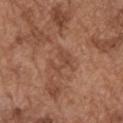notes: imaged on a skin check; not biopsied | imaging modality: total-body-photography crop, ~15 mm field of view | location: the right upper arm | subject: male, aged approximately 75.